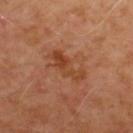notes: total-body-photography surveillance lesion; no biopsy
automated lesion analysis: about 8 CIELAB-L* units darker than the surrounding skin
anatomic site: the upper back
imaging modality: 15 mm crop, total-body photography
patient: male, roughly 50 years of age
diameter: ≈5 mm